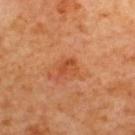  biopsy_status: not biopsied; imaged during a skin examination
  image:
    source: total-body photography crop
    field_of_view_mm: 15
  site: upper back
  patient:
    sex: female
    age_approx: 65